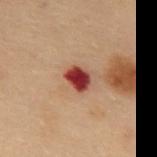Assessment: This lesion was catalogued during total-body skin photography and was not selected for biopsy. Clinical summary: Automated image analysis of the tile measured about 17 CIELAB-L* units darker than the surrounding skin and a normalized border contrast of about 14.5. A 15 mm close-up extracted from a 3D total-body photography capture. Approximately 3 mm at its widest. This is a cross-polarized tile. A male subject roughly 55 years of age. The lesion is located on the abdomen.